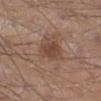Q: Was this lesion biopsied?
A: imaged on a skin check; not biopsied
Q: Patient demographics?
A: male, approximately 30 years of age
Q: How was this image acquired?
A: ~15 mm tile from a whole-body skin photo
Q: Where on the body is the lesion?
A: the left lower leg